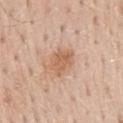Clinical impression: Recorded during total-body skin imaging; not selected for excision or biopsy. Context: Longest diameter approximately 3.5 mm. This is a white-light tile. Automated image analysis of the tile measured an area of roughly 8 mm² and an eccentricity of roughly 0.7. The analysis additionally found border irregularity of about 2.5 on a 0–10 scale, a within-lesion color-variation index near 2.5/10, and peripheral color asymmetry of about 1. The lesion is located on the mid back. A male patient aged 58 to 62. Cropped from a total-body skin-imaging series; the visible field is about 15 mm.